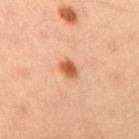Case summary:
- follow-up · catalogued during a skin exam; not biopsied
- image-analysis metrics · a lesion color around L≈59 a*≈28 b*≈40 in CIELAB and a lesion–skin lightness drop of about 14; a classifier nevus-likeness of about 100/100 and a lesion-detection confidence of about 100/100
- acquisition · ~15 mm crop, total-body skin-cancer survey
- anatomic site · the right upper arm
- diameter · about 2.5 mm
- subject · female, aged 33 to 37